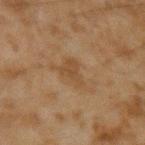lighting = cross-polarized illumination
subject = male, aged approximately 45
imaging modality = 15 mm crop, total-body photography
image-analysis metrics = a lesion area of about 5 mm² and two-axis asymmetry of about 0.5; an average lesion color of about L≈37 a*≈15 b*≈29 (CIELAB), a lesion–skin lightness drop of about 5, and a normalized lesion–skin contrast near 5.5; a border-irregularity rating of about 5/10, internal color variation of about 1 on a 0–10 scale, and radial color variation of about 0.5
body site = the arm
size = ≈3 mm